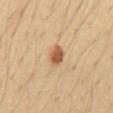Q: How was the tile lit?
A: cross-polarized illumination
Q: Where on the body is the lesion?
A: the abdomen
Q: How was this image acquired?
A: ~15 mm crop, total-body skin-cancer survey
Q: What is the lesion's diameter?
A: about 2.5 mm
Q: Automated lesion metrics?
A: a symmetry-axis asymmetry near 0.25
Q: Patient demographics?
A: male, approximately 35 years of age